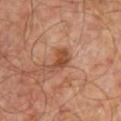Imaged during a routine full-body skin examination; the lesion was not biopsied and no histopathology is available. Longest diameter approximately 3.5 mm. A lesion tile, about 15 mm wide, cut from a 3D total-body photograph. The subject is a male approximately 55 years of age. The lesion is located on the front of the torso.